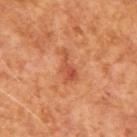Assessment:
The lesion was photographed on a routine skin check and not biopsied; there is no pathology result.
Background:
The tile uses cross-polarized illumination. About 3.5 mm across. This image is a 15 mm lesion crop taken from a total-body photograph. A male subject, aged 63–67. Automated tile analysis of the lesion measured an area of roughly 4 mm², an outline eccentricity of about 0.9 (0 = round, 1 = elongated), and two-axis asymmetry of about 0.6.